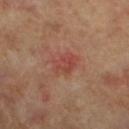<tbp_lesion>
<biopsy_status>not biopsied; imaged during a skin examination</biopsy_status>
<site>right leg</site>
<lighting>cross-polarized</lighting>
<lesion_size>
  <long_diameter_mm_approx>3.0</long_diameter_mm_approx>
</lesion_size>
<patient>
  <sex>female</sex>
  <age_approx>65</age_approx>
</patient>
<image>
  <source>total-body photography crop</source>
  <field_of_view_mm>15</field_of_view_mm>
</image>
</tbp_lesion>